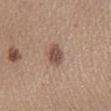Imaged during a routine full-body skin examination; the lesion was not biopsied and no histopathology is available.
The lesion is located on the right lower leg.
A 15 mm close-up tile from a total-body photography series done for melanoma screening.
An algorithmic analysis of the crop reported an average lesion color of about L≈50 a*≈18 b*≈25 (CIELAB), about 12 CIELAB-L* units darker than the surrounding skin, and a lesion-to-skin contrast of about 8.5 (normalized; higher = more distinct).
Captured under white-light illumination.
The subject is a male approximately 35 years of age.
Longest diameter approximately 3 mm.